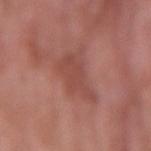Impression:
The lesion was photographed on a routine skin check and not biopsied; there is no pathology result.
Clinical summary:
The tile uses white-light illumination. On the left upper arm. A 15 mm close-up extracted from a 3D total-body photography capture. The recorded lesion diameter is about 5.5 mm. The subject is a female aged around 70.